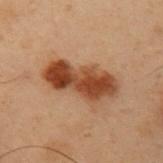follow-up: no biopsy performed (imaged during a skin exam) | patient: male, aged 53–57 | image source: 15 mm crop, total-body photography | automated lesion analysis: an area of roughly 20 mm², an outline eccentricity of about 0.9 (0 = round, 1 = elongated), and a symmetry-axis asymmetry near 0.25; a mean CIELAB color near L≈36 a*≈20 b*≈29; a nevus-likeness score of about 100/100 and a detector confidence of about 100 out of 100 that the crop contains a lesion | anatomic site: the left upper arm | size: ≈7 mm.